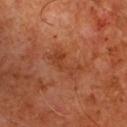<record>
<biopsy_status>not biopsied; imaged during a skin examination</biopsy_status>
<patient>
  <sex>male</sex>
  <age_approx>60</age_approx>
</patient>
<site>front of the torso</site>
<automated_metrics>
  <cielab_L>40</cielab_L>
  <cielab_a>27</cielab_a>
  <cielab_b>35</cielab_b>
  <vs_skin_darker_L>6.0</vs_skin_darker_L>
  <vs_skin_contrast_norm>5.5</vs_skin_contrast_norm>
</automated_metrics>
<image>
  <source>total-body photography crop</source>
  <field_of_view_mm>15</field_of_view_mm>
</image>
<lighting>cross-polarized</lighting>
</record>